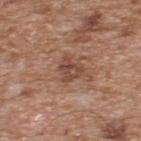workup = catalogued during a skin exam; not biopsied | diameter = ~3 mm (longest diameter) | patient = male, about 65 years old | TBP lesion metrics = a lesion color around L≈46 a*≈21 b*≈27 in CIELAB and about 9 CIELAB-L* units darker than the surrounding skin; a color-variation rating of about 5.5/10 and radial color variation of about 2.5 | acquisition = 15 mm crop, total-body photography | anatomic site = the back | tile lighting = white-light illumination.